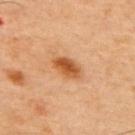{
  "biopsy_status": "not biopsied; imaged during a skin examination",
  "lighting": "cross-polarized",
  "site": "upper back",
  "automated_metrics": {
    "cielab_L": 52,
    "cielab_a": 25,
    "cielab_b": 41,
    "vs_skin_darker_L": 12.0,
    "vs_skin_contrast_norm": 9.5,
    "border_irregularity_0_10": 2.0,
    "color_variation_0_10": 4.0,
    "peripheral_color_asymmetry": 1.5
  },
  "patient": {
    "sex": "male",
    "age_approx": 45
  },
  "image": {
    "source": "total-body photography crop",
    "field_of_view_mm": 15
  },
  "lesion_size": {
    "long_diameter_mm_approx": 4.0
  }
}